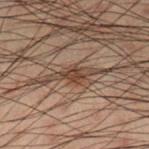notes: catalogued during a skin exam; not biopsied | image source: ~15 mm crop, total-body skin-cancer survey | automated lesion analysis: a footprint of about 6 mm², an outline eccentricity of about 0.95 (0 = round, 1 = elongated), and a symmetry-axis asymmetry near 0.25; a lesion-to-skin contrast of about 8 (normalized; higher = more distinct); a border-irregularity rating of about 4.5/10 and internal color variation of about 1.5 on a 0–10 scale; an automated nevus-likeness rating near 50 out of 100 and a lesion-detection confidence of about 55/100 | lesion diameter: ~5 mm (longest diameter) | patient: male, aged approximately 50 | site: the leg | lighting: cross-polarized illumination.